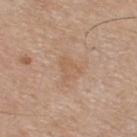Findings:
* workup — total-body-photography surveillance lesion; no biopsy
* body site — the upper back
* image — ~15 mm crop, total-body skin-cancer survey
* tile lighting — white-light illumination
* subject — male, in their mid-70s
* image-analysis metrics — roughly 5 lightness units darker than nearby skin and a lesion-to-skin contrast of about 4.5 (normalized; higher = more distinct); a within-lesion color-variation index near 0.5/10